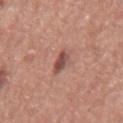{"biopsy_status": "not biopsied; imaged during a skin examination", "lighting": "white-light", "lesion_size": {"long_diameter_mm_approx": 2.5}, "image": {"source": "total-body photography crop", "field_of_view_mm": 15}, "patient": {"sex": "male", "age_approx": 75}, "site": "mid back", "automated_metrics": {"vs_skin_contrast_norm": 9.0, "nevus_likeness_0_100": 75, "lesion_detection_confidence_0_100": 100}}